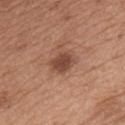{
  "biopsy_status": "not biopsied; imaged during a skin examination",
  "patient": {
    "sex": "male",
    "age_approx": 65
  },
  "lighting": "white-light",
  "automated_metrics": {
    "area_mm2_approx": 6.5,
    "eccentricity": 0.7,
    "shape_asymmetry": 0.15,
    "cielab_L": 46,
    "cielab_a": 22,
    "cielab_b": 28,
    "vs_skin_darker_L": 11.0,
    "vs_skin_contrast_norm": 8.5
  },
  "lesion_size": {
    "long_diameter_mm_approx": 3.5
  },
  "image": {
    "source": "total-body photography crop",
    "field_of_view_mm": 15
  },
  "site": "chest"
}